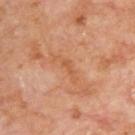biopsy status = imaged on a skin check; not biopsied | body site = the upper back | imaging modality = ~15 mm crop, total-body skin-cancer survey | illumination = cross-polarized illumination | patient = male, aged around 70 | lesion diameter = ~3.5 mm (longest diameter).